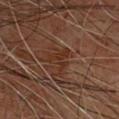Q: Is there a histopathology result?
A: no biopsy performed (imaged during a skin exam)
Q: How was the tile lit?
A: cross-polarized illumination
Q: Who is the patient?
A: male, aged around 60
Q: Where on the body is the lesion?
A: the back
Q: Lesion size?
A: about 3.5 mm
Q: Automated lesion metrics?
A: a normalized lesion–skin contrast near 6.5; a within-lesion color-variation index near 2/10 and peripheral color asymmetry of about 0.5; a nevus-likeness score of about 0/100
Q: How was this image acquired?
A: ~15 mm tile from a whole-body skin photo